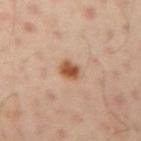Findings:
• workup — imaged on a skin check; not biopsied
• image source — ~15 mm tile from a whole-body skin photo
• lighting — cross-polarized illumination
• location — the left arm
• patient — male, roughly 50 years of age
• size — ~2.5 mm (longest diameter)
• image-analysis metrics — a lesion area of about 4.5 mm², an outline eccentricity of about 0.55 (0 = round, 1 = elongated), and a symmetry-axis asymmetry near 0.2; a lesion–skin lightness drop of about 13 and a normalized border contrast of about 10